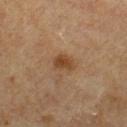Q: Was this lesion biopsied?
A: no biopsy performed (imaged during a skin exam)
Q: What did automated image analysis measure?
A: a footprint of about 4.5 mm², an eccentricity of roughly 0.65, and two-axis asymmetry of about 0.3; a lesion color around L≈39 a*≈17 b*≈31 in CIELAB and a lesion-to-skin contrast of about 8 (normalized; higher = more distinct); a border-irregularity index near 2.5/10, a within-lesion color-variation index near 2.5/10, and peripheral color asymmetry of about 1; lesion-presence confidence of about 100/100
Q: What is the lesion's diameter?
A: about 2.5 mm
Q: Lesion location?
A: the chest
Q: What kind of image is this?
A: 15 mm crop, total-body photography
Q: Patient demographics?
A: male, about 65 years old